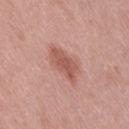{"biopsy_status": "not biopsied; imaged during a skin examination", "site": "right thigh", "automated_metrics": {"area_mm2_approx": 9.0, "eccentricity": 0.85, "border_irregularity_0_10": 3.5, "color_variation_0_10": 2.5, "peripheral_color_asymmetry": 1.0}, "lighting": "white-light", "image": {"source": "total-body photography crop", "field_of_view_mm": 15}, "patient": {"sex": "female", "age_approx": 40}}